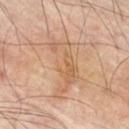<record>
  <biopsy_status>not biopsied; imaged during a skin examination</biopsy_status>
  <image>
    <source>total-body photography crop</source>
    <field_of_view_mm>15</field_of_view_mm>
  </image>
  <site>right thigh</site>
  <patient>
    <sex>male</sex>
    <age_approx>70</age_approx>
  </patient>
</record>